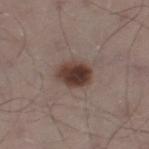biopsy status: no biopsy performed (imaged during a skin exam); site: the left thigh; subject: male, approximately 55 years of age; acquisition: 15 mm crop, total-body photography; lesion size: about 4 mm; lighting: white-light illumination.